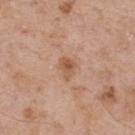Part of a total-body skin-imaging series; this lesion was reviewed on a skin check and was not flagged for biopsy.